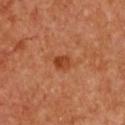Recorded during total-body skin imaging; not selected for excision or biopsy. From the chest. Captured under cross-polarized illumination. The total-body-photography lesion software estimated an area of roughly 3.5 mm² and a symmetry-axis asymmetry near 0.25. It also reported a within-lesion color-variation index near 4.5/10 and peripheral color asymmetry of about 2. About 2 mm across. The patient is a female aged approximately 65. A roughly 15 mm field-of-view crop from a total-body skin photograph.